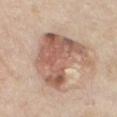follow-up=no biopsy performed (imaged during a skin exam) | anatomic site=the chest | image=15 mm crop, total-body photography | patient=male, in their 60s | tile lighting=white-light | diameter=about 7 mm.